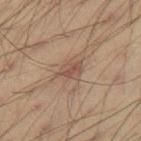notes: total-body-photography surveillance lesion; no biopsy
diameter: about 3 mm
acquisition: ~15 mm tile from a whole-body skin photo
tile lighting: cross-polarized
location: the right thigh
subject: male, approximately 40 years of age
automated lesion analysis: an outline eccentricity of about 0.75 (0 = round, 1 = elongated) and two-axis asymmetry of about 0.3; a lesion-detection confidence of about 100/100